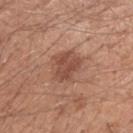A male patient aged approximately 65. The lesion is located on the left upper arm. Imaged with white-light lighting. A 15 mm crop from a total-body photograph taken for skin-cancer surveillance.